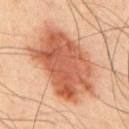{"biopsy_status": "not biopsied; imaged during a skin examination", "site": "upper back", "image": {"source": "total-body photography crop", "field_of_view_mm": 15}, "patient": {"sex": "male", "age_approx": 50}, "lighting": "cross-polarized", "lesion_size": {"long_diameter_mm_approx": 10.0}, "automated_metrics": {"area_mm2_approx": 44.0, "eccentricity": 0.8, "shape_asymmetry": 0.2}}